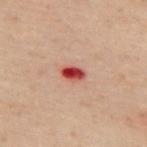This lesion was catalogued during total-body skin photography and was not selected for biopsy.
Cropped from a whole-body photographic skin survey; the tile spans about 15 mm.
The lesion is located on the upper back.
A male patient, aged 48–52.
Captured under cross-polarized illumination.
The lesion's longest dimension is about 2.5 mm.